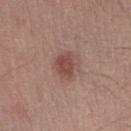Notes:
– biopsy status — total-body-photography surveillance lesion; no biopsy
– acquisition — total-body-photography crop, ~15 mm field of view
– TBP lesion metrics — a lesion area of about 6 mm², an outline eccentricity of about 0.65 (0 = round, 1 = elongated), and a shape-asymmetry score of about 0.3 (0 = symmetric); a lesion color around L≈47 a*≈21 b*≈23 in CIELAB, a lesion–skin lightness drop of about 10, and a normalized lesion–skin contrast near 7.5; an automated nevus-likeness rating near 90 out of 100
– tile lighting — white-light illumination
– body site — the right lower leg
– lesion diameter — about 3 mm
– patient — male, about 45 years old